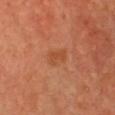Assessment:
The lesion was tiled from a total-body skin photograph and was not biopsied.
Clinical summary:
A lesion tile, about 15 mm wide, cut from a 3D total-body photograph. Automated image analysis of the tile measured a footprint of about 4 mm², an eccentricity of roughly 0.8, and a symmetry-axis asymmetry near 0.3. The software also gave a mean CIELAB color near L≈48 a*≈27 b*≈38, roughly 6 lightness units darker than nearby skin, and a lesion-to-skin contrast of about 5.5 (normalized; higher = more distinct). The analysis additionally found border irregularity of about 3 on a 0–10 scale, internal color variation of about 1.5 on a 0–10 scale, and peripheral color asymmetry of about 0.5. This is a cross-polarized tile. Longest diameter approximately 3 mm. The patient is a female aged 43–47. From the chest.